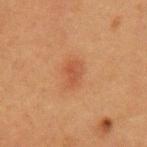Part of a total-body skin-imaging series; this lesion was reviewed on a skin check and was not flagged for biopsy.
The lesion is located on the arm.
A roughly 15 mm field-of-view crop from a total-body skin photograph.
A female patient, in their 40s.